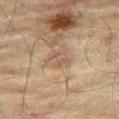Imaged during a routine full-body skin examination; the lesion was not biopsied and no histopathology is available. The lesion is located on the leg. A male patient in their 70s. About 2.5 mm across. This is a cross-polarized tile. The total-body-photography lesion software estimated a border-irregularity rating of about 4/10 and radial color variation of about 1. It also reported a detector confidence of about 60 out of 100 that the crop contains a lesion. A region of skin cropped from a whole-body photographic capture, roughly 15 mm wide.